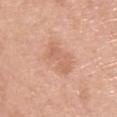Assessment:
Captured during whole-body skin photography for melanoma surveillance; the lesion was not biopsied.
Image and clinical context:
This image is a 15 mm lesion crop taken from a total-body photograph. The subject is a male aged approximately 55. Automated tile analysis of the lesion measured an outline eccentricity of about 0.65 (0 = round, 1 = elongated) and a symmetry-axis asymmetry near 0.2. The software also gave a border-irregularity index near 3.5/10, a color-variation rating of about 2.5/10, and peripheral color asymmetry of about 0.5. Measured at roughly 4 mm in maximum diameter. On the back. The tile uses white-light illumination.